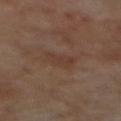The lesion was tiled from a total-body skin photograph and was not biopsied. A male subject aged 68 to 72. The lesion is located on the mid back. The tile uses cross-polarized illumination. Cropped from a total-body skin-imaging series; the visible field is about 15 mm.